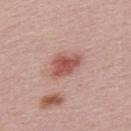notes: imaged on a skin check; not biopsied | tile lighting: white-light | automated lesion analysis: a mean CIELAB color near L≈53 a*≈27 b*≈25, roughly 12 lightness units darker than nearby skin, and a normalized lesion–skin contrast near 8.5 | size: ≈4 mm | image: total-body-photography crop, ~15 mm field of view | subject: male, aged around 30.